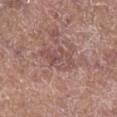automated lesion analysis: an outline eccentricity of about 0.85 (0 = round, 1 = elongated) and a symmetry-axis asymmetry near 0.3; border irregularity of about 3.5 on a 0–10 scale, internal color variation of about 4.5 on a 0–10 scale, and a peripheral color-asymmetry measure near 1.5
subject: male, aged 68 to 72
location: the right lower leg
illumination: white-light illumination
lesion size: about 4.5 mm
imaging modality: ~15 mm tile from a whole-body skin photo